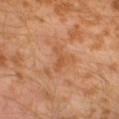Impression: The lesion was tiled from a total-body skin photograph and was not biopsied. Clinical summary: Cropped from a whole-body photographic skin survey; the tile spans about 15 mm. About 3.5 mm across. On the left lower leg. Captured under cross-polarized illumination. A male patient aged around 30.